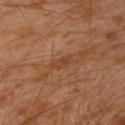biopsy status: no biopsy performed (imaged during a skin exam) | automated lesion analysis: a lesion–skin lightness drop of about 6 and a lesion-to-skin contrast of about 5.5 (normalized; higher = more distinct); an automated nevus-likeness rating near 0 out of 100 | image: total-body-photography crop, ~15 mm field of view | anatomic site: the left upper arm | lesion diameter: about 2.5 mm | illumination: cross-polarized illumination | patient: male, aged approximately 30.